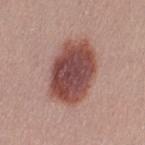Recorded during total-body skin imaging; not selected for excision or biopsy. The lesion is located on the left thigh. Measured at roughly 7 mm in maximum diameter. Cropped from a whole-body photographic skin survey; the tile spans about 15 mm. The subject is a female about 25 years old.